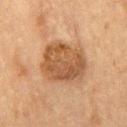Notes:
* follow-up — imaged on a skin check; not biopsied
* image-analysis metrics — a footprint of about 21 mm² and a shape-asymmetry score of about 0.2 (0 = symmetric); a mean CIELAB color near L≈43 a*≈18 b*≈31, a lesion–skin lightness drop of about 10, and a normalized border contrast of about 8
* tile lighting — cross-polarized illumination
* subject — male, in their mid-70s
* image — ~15 mm tile from a whole-body skin photo
* diameter — ≈6 mm
* site — the chest